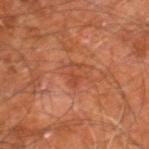Image and clinical context:
The subject is a male roughly 60 years of age. About 2.5 mm across. A 15 mm close-up tile from a total-body photography series done for melanoma screening. Located on the right leg.